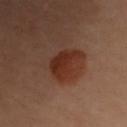Q: How large is the lesion?
A: ~3.5 mm (longest diameter)
Q: How was the tile lit?
A: cross-polarized illumination
Q: What did automated image analysis measure?
A: a lesion color around L≈25 a*≈20 b*≈25 in CIELAB and a normalized border contrast of about 9.5
Q: Patient demographics?
A: female, aged approximately 60
Q: What is the imaging modality?
A: ~15 mm crop, total-body skin-cancer survey
Q: What is the anatomic site?
A: the right arm